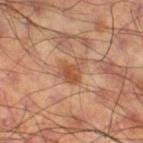Case summary:
- notes: total-body-photography surveillance lesion; no biopsy
- automated metrics: border irregularity of about 5 on a 0–10 scale, a within-lesion color-variation index near 3.5/10, and peripheral color asymmetry of about 1
- image: total-body-photography crop, ~15 mm field of view
- subject: male, aged approximately 60
- lesion size: ≈3 mm
- tile lighting: cross-polarized illumination
- anatomic site: the right thigh